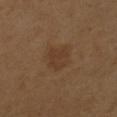| field | value |
|---|---|
| notes | imaged on a skin check; not biopsied |
| body site | the right forearm |
| acquisition | ~15 mm tile from a whole-body skin photo |
| TBP lesion metrics | a lesion color around L≈37 a*≈17 b*≈30 in CIELAB and roughly 5 lightness units darker than nearby skin; a within-lesion color-variation index near 2/10 and radial color variation of about 0.5; an automated nevus-likeness rating near 5 out of 100 |
| subject | female, aged around 55 |
| lesion size | ~3.5 mm (longest diameter) |